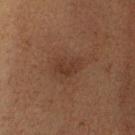This lesion was catalogued during total-body skin photography and was not selected for biopsy.